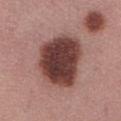Impression:
Part of a total-body skin-imaging series; this lesion was reviewed on a skin check and was not flagged for biopsy.
Image and clinical context:
The lesion is located on the abdomen. A female subject about 55 years old. The recorded lesion diameter is about 7 mm. Automated tile analysis of the lesion measured a nevus-likeness score of about 10/100 and a lesion-detection confidence of about 100/100. A roughly 15 mm field-of-view crop from a total-body skin photograph.